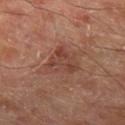| key | value |
|---|---|
| follow-up | imaged on a skin check; not biopsied |
| location | the left thigh |
| patient | male, aged 68–72 |
| image-analysis metrics | an average lesion color of about L≈42 a*≈22 b*≈26 (CIELAB); a within-lesion color-variation index near 4.5/10 and peripheral color asymmetry of about 1.5 |
| image source | total-body-photography crop, ~15 mm field of view |
| illumination | cross-polarized |